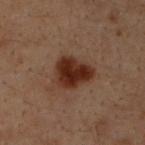| field | value |
|---|---|
| notes | no biopsy performed (imaged during a skin exam) |
| location | the upper back |
| lighting | cross-polarized illumination |
| lesion diameter | ~4.5 mm (longest diameter) |
| acquisition | ~15 mm tile from a whole-body skin photo |
| subject | male, aged 28 to 32 |